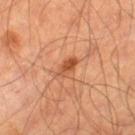Findings:
- workup: total-body-photography surveillance lesion; no biopsy
- subject: male, aged around 65
- imaging modality: ~15 mm crop, total-body skin-cancer survey
- automated lesion analysis: a lesion area of about 3.5 mm² and a shape-asymmetry score of about 0.3 (0 = symmetric); an average lesion color of about L≈48 a*≈25 b*≈36 (CIELAB), a lesion–skin lightness drop of about 10, and a normalized lesion–skin contrast near 7.5; a border-irregularity index near 3/10 and a within-lesion color-variation index near 4/10; a nevus-likeness score of about 85/100 and lesion-presence confidence of about 100/100
- body site: the right thigh
- tile lighting: cross-polarized
- diameter: ~3 mm (longest diameter)